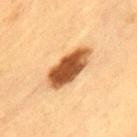Impression:
The lesion was tiled from a total-body skin photograph and was not biopsied.
Acquisition and patient details:
A female subject aged around 40. A close-up tile cropped from a whole-body skin photograph, about 15 mm across. From the left thigh. About 6.5 mm across. The total-body-photography lesion software estimated a footprint of about 15 mm², an eccentricity of roughly 0.9, and two-axis asymmetry of about 0.15. The analysis additionally found a lesion color around L≈48 a*≈22 b*≈37 in CIELAB, a lesion–skin lightness drop of about 20, and a lesion-to-skin contrast of about 13.5 (normalized; higher = more distinct). The analysis additionally found an automated nevus-likeness rating near 100 out of 100 and a lesion-detection confidence of about 100/100. Captured under cross-polarized illumination.